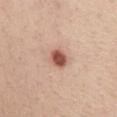Case summary:
• follow-up — total-body-photography surveillance lesion; no biopsy
• patient — female, in their 50s
• anatomic site — the chest
• illumination — white-light
• acquisition — 15 mm crop, total-body photography
• diameter — ~2.5 mm (longest diameter)